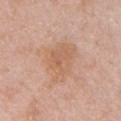Findings:
- follow-up — catalogued during a skin exam; not biopsied